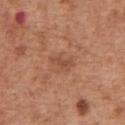Q: Was a biopsy performed?
A: catalogued during a skin exam; not biopsied
Q: Lesion size?
A: ≈3 mm
Q: What is the imaging modality?
A: total-body-photography crop, ~15 mm field of view
Q: Where on the body is the lesion?
A: the abdomen
Q: What are the patient's age and sex?
A: male, aged 63–67
Q: Illumination type?
A: white-light